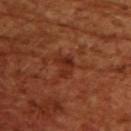No biopsy was performed on this lesion — it was imaged during a full skin examination and was not determined to be concerning.
About 3 mm across.
The total-body-photography lesion software estimated a lesion area of about 4.5 mm², a shape eccentricity near 0.65, and a shape-asymmetry score of about 0.4 (0 = symmetric). The analysis additionally found a mean CIELAB color near L≈31 a*≈28 b*≈32, about 7 CIELAB-L* units darker than the surrounding skin, and a normalized lesion–skin contrast near 6.5. It also reported a classifier nevus-likeness of about 0/100 and lesion-presence confidence of about 100/100.
On the upper back.
A female subject, about 55 years old.
This image is a 15 mm lesion crop taken from a total-body photograph.
This is a cross-polarized tile.